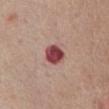workup: imaged on a skin check; not biopsied | site: the abdomen | subject: female, aged approximately 65 | image: 15 mm crop, total-body photography.